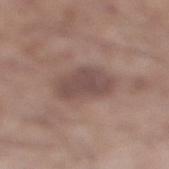Part of a total-body skin-imaging series; this lesion was reviewed on a skin check and was not flagged for biopsy.
The lesion-visualizer software estimated a footprint of about 10 mm², an eccentricity of roughly 0.75, and two-axis asymmetry of about 0.25. It also reported a border-irregularity index near 2.5/10 and peripheral color asymmetry of about 0.5.
The tile uses white-light illumination.
A lesion tile, about 15 mm wide, cut from a 3D total-body photograph.
The lesion is on the right lower leg.
The patient is a male in their mid- to late 50s.
Longest diameter approximately 4.5 mm.